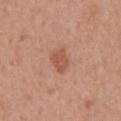Case summary:
– subject: male, about 70 years old
– site: the chest
– image: ~15 mm tile from a whole-body skin photo